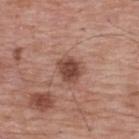  biopsy_status: not biopsied; imaged during a skin examination
  lesion_size:
    long_diameter_mm_approx: 3.0
  patient:
    sex: male
    age_approx: 70
  lighting: white-light
  site: upper back
  image:
    source: total-body photography crop
    field_of_view_mm: 15